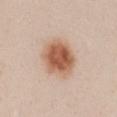  biopsy_status: not biopsied; imaged during a skin examination
  patient:
    sex: female
    age_approx: 25
  site: front of the torso
  automated_metrics:
    area_mm2_approx: 15.0
    eccentricity: 0.4
    shape_asymmetry: 0.1
  lesion_size:
    long_diameter_mm_approx: 4.5
  lighting: white-light
  image:
    source: total-body photography crop
    field_of_view_mm: 15